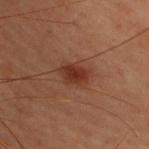workup = catalogued during a skin exam; not biopsied | size = ~3.5 mm (longest diameter) | lighting = cross-polarized | image-analysis metrics = an area of roughly 5.5 mm² and an eccentricity of roughly 0.75; a color-variation rating of about 3/10 and peripheral color asymmetry of about 1 | location = the upper back | image source = total-body-photography crop, ~15 mm field of view | subject = female, aged 38–42.